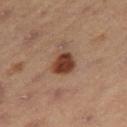Notes:
* acquisition — ~15 mm crop, total-body skin-cancer survey
* lesion diameter — ≈3.5 mm
* subject — male, in their mid- to late 50s
* tile lighting — cross-polarized illumination
* site — the right thigh